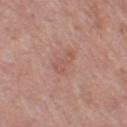Imaged during a routine full-body skin examination; the lesion was not biopsied and no histopathology is available.
The lesion is located on the left thigh.
The patient is a female aged around 50.
About 3 mm across.
Cropped from a whole-body photographic skin survey; the tile spans about 15 mm.
This is a white-light tile.